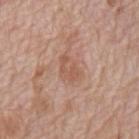biopsy_status: not biopsied; imaged during a skin examination
lighting: white-light
image:
  source: total-body photography crop
  field_of_view_mm: 15
lesion_size:
  long_diameter_mm_approx: 3.0
patient:
  sex: male
  age_approx: 70
automated_metrics:
  area_mm2_approx: 4.5
  shape_asymmetry: 0.45
  cielab_L: 55
  cielab_a: 21
  cielab_b: 30
  vs_skin_darker_L: 8.0
  vs_skin_contrast_norm: 6.0
  border_irregularity_0_10: 6.0
  color_variation_0_10: 1.0
site: mid back